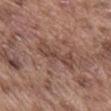Notes:
– workup — imaged on a skin check; not biopsied
– subject — male, aged 73–77
– lesion size — ~5 mm (longest diameter)
– image-analysis metrics — a mean CIELAB color near L≈45 a*≈19 b*≈24, about 8 CIELAB-L* units darker than the surrounding skin, and a normalized border contrast of about 6; a border-irregularity rating of about 6/10; a classifier nevus-likeness of about 0/100 and a detector confidence of about 65 out of 100 that the crop contains a lesion
– body site — the abdomen
– image — 15 mm crop, total-body photography